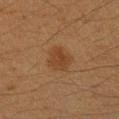Q: Is there a histopathology result?
A: no biopsy performed (imaged during a skin exam)
Q: How was this image acquired?
A: ~15 mm crop, total-body skin-cancer survey
Q: What are the patient's age and sex?
A: female, roughly 40 years of age
Q: Illumination type?
A: cross-polarized illumination
Q: How large is the lesion?
A: about 3 mm
Q: Where on the body is the lesion?
A: the left forearm